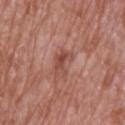follow-up=imaged on a skin check; not biopsied | lesion diameter=≈3 mm | acquisition=~15 mm crop, total-body skin-cancer survey | lighting=white-light illumination | patient=male, aged around 70 | anatomic site=the upper back.